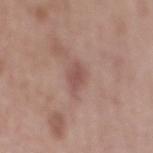The recorded lesion diameter is about 3 mm.
The lesion is on the mid back.
Captured under white-light illumination.
A 15 mm close-up extracted from a 3D total-body photography capture.
A male patient, aged around 65.